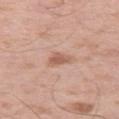Clinical impression:
Part of a total-body skin-imaging series; this lesion was reviewed on a skin check and was not flagged for biopsy.
Image and clinical context:
A region of skin cropped from a whole-body photographic capture, roughly 15 mm wide. About 2.5 mm across. Imaged with white-light lighting. A male patient, aged 58 to 62. The lesion is located on the right thigh.